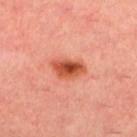biopsy_status: not biopsied; imaged during a skin examination
site: left lower leg
lighting: cross-polarized
lesion_size:
  long_diameter_mm_approx: 4.0
patient:
  sex: female
  age_approx: 35
image:
  source: total-body photography crop
  field_of_view_mm: 15